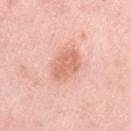follow-up=no biopsy performed (imaged during a skin exam); image source=~15 mm crop, total-body skin-cancer survey; size=~4.5 mm (longest diameter); subject=female, aged approximately 65; body site=the left upper arm; illumination=white-light illumination.